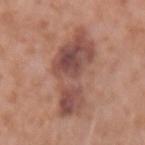Part of a total-body skin-imaging series; this lesion was reviewed on a skin check and was not flagged for biopsy.
The recorded lesion diameter is about 8.5 mm.
The lesion is on the right forearm.
A region of skin cropped from a whole-body photographic capture, roughly 15 mm wide.
The tile uses white-light illumination.
Automated tile analysis of the lesion measured a footprint of about 27 mm², an eccentricity of roughly 0.85, and a symmetry-axis asymmetry near 0.4. The analysis additionally found an average lesion color of about L≈49 a*≈22 b*≈25 (CIELAB) and a normalized lesion–skin contrast near 8.5. It also reported a border-irregularity index near 5/10, a within-lesion color-variation index near 7.5/10, and peripheral color asymmetry of about 3.
A female subject, aged 38–42.